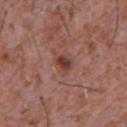Captured during whole-body skin photography for melanoma surveillance; the lesion was not biopsied.
The lesion-visualizer software estimated a footprint of about 4.5 mm² and two-axis asymmetry of about 0.35. It also reported a lesion color around L≈42 a*≈23 b*≈26 in CIELAB, roughly 9 lightness units darker than nearby skin, and a normalized border contrast of about 8. The software also gave border irregularity of about 3 on a 0–10 scale and peripheral color asymmetry of about 2. It also reported a lesion-detection confidence of about 100/100.
The recorded lesion diameter is about 3 mm.
This is a white-light tile.
A male subject aged 43–47.
A 15 mm close-up tile from a total-body photography series done for melanoma screening.
Located on the chest.